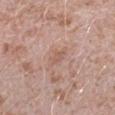Recorded during total-body skin imaging; not selected for excision or biopsy.
Automated tile analysis of the lesion measured an average lesion color of about L≈58 a*≈20 b*≈26 (CIELAB) and a lesion-to-skin contrast of about 5 (normalized; higher = more distinct). It also reported border irregularity of about 4 on a 0–10 scale, a color-variation rating of about 1/10, and radial color variation of about 0.5. It also reported an automated nevus-likeness rating near 0 out of 100 and a detector confidence of about 100 out of 100 that the crop contains a lesion.
A 15 mm close-up extracted from a 3D total-body photography capture.
A male patient roughly 40 years of age.
About 3 mm across.
Located on the arm.
The tile uses white-light illumination.